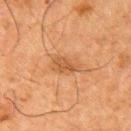<record>
<biopsy_status>not biopsied; imaged during a skin examination</biopsy_status>
<site>arm</site>
<patient>
  <sex>male</sex>
  <age_approx>80</age_approx>
</patient>
<lighting>cross-polarized</lighting>
<automated_metrics>
  <cielab_L>44</cielab_L>
  <cielab_a>20</cielab_a>
  <cielab_b>33</cielab_b>
  <vs_skin_darker_L>7.0</vs_skin_darker_L>
  <vs_skin_contrast_norm>6.0</vs_skin_contrast_norm>
  <border_irregularity_0_10>3.0</border_irregularity_0_10>
  <color_variation_0_10>2.0</color_variation_0_10>
  <peripheral_color_asymmetry>0.5</peripheral_color_asymmetry>
</automated_metrics>
<lesion_size>
  <long_diameter_mm_approx>3.0</long_diameter_mm_approx>
</lesion_size>
<image>
  <source>total-body photography crop</source>
  <field_of_view_mm>15</field_of_view_mm>
</image>
</record>